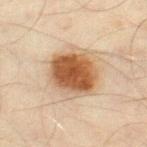<lesion>
  <biopsy_status>not biopsied; imaged during a skin examination</biopsy_status>
  <patient>
    <sex>male</sex>
    <age_approx>45</age_approx>
  </patient>
  <lighting>cross-polarized</lighting>
  <lesion_size>
    <long_diameter_mm_approx>5.5</long_diameter_mm_approx>
  </lesion_size>
  <image>
    <source>total-body photography crop</source>
    <field_of_view_mm>15</field_of_view_mm>
  </image>
  <site>right thigh</site>
</lesion>